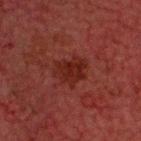Clinical impression: Imaged during a routine full-body skin examination; the lesion was not biopsied and no histopathology is available. Background: The lesion is on the head or neck. Approximately 4 mm at its widest. A roughly 15 mm field-of-view crop from a total-body skin photograph. The tile uses cross-polarized illumination. The total-body-photography lesion software estimated a lesion area of about 9 mm² and a symmetry-axis asymmetry near 0.3. The analysis additionally found a mean CIELAB color near L≈19 a*≈23 b*≈21, roughly 6 lightness units darker than nearby skin, and a normalized border contrast of about 7.5. The analysis additionally found border irregularity of about 3.5 on a 0–10 scale, a within-lesion color-variation index near 2/10, and radial color variation of about 1. A male subject roughly 60 years of age.